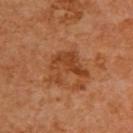<case>
  <biopsy_status>not biopsied; imaged during a skin examination</biopsy_status>
  <site>upper back</site>
  <patient>
    <sex>male</sex>
    <age_approx>60</age_approx>
  </patient>
  <lighting>cross-polarized</lighting>
  <image>
    <source>total-body photography crop</source>
    <field_of_view_mm>15</field_of_view_mm>
  </image>
  <lesion_size>
    <long_diameter_mm_approx>5.0</long_diameter_mm_approx>
  </lesion_size>
</case>